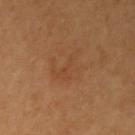Q: Was a biopsy performed?
A: catalogued during a skin exam; not biopsied
Q: What are the patient's age and sex?
A: female, in their 60s
Q: Automated lesion metrics?
A: a lesion area of about 8.5 mm², an outline eccentricity of about 0.7 (0 = round, 1 = elongated), and a shape-asymmetry score of about 0.65 (0 = symmetric); an automated nevus-likeness rating near 0 out of 100 and lesion-presence confidence of about 100/100
Q: What kind of image is this?
A: ~15 mm tile from a whole-body skin photo
Q: What is the lesion's diameter?
A: ~4.5 mm (longest diameter)
Q: What is the anatomic site?
A: the left arm